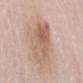{"biopsy_status": "not biopsied; imaged during a skin examination", "patient": {"sex": "male", "age_approx": 65}, "lighting": "white-light", "lesion_size": {"long_diameter_mm_approx": 7.0}, "image": {"source": "total-body photography crop", "field_of_view_mm": 15}, "site": "mid back", "automated_metrics": {"cielab_L": 60, "cielab_a": 18, "cielab_b": 28, "vs_skin_darker_L": 9.0, "vs_skin_contrast_norm": 6.5, "lesion_detection_confidence_0_100": 100}}